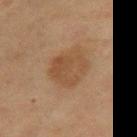biopsy status — no biopsy performed (imaged during a skin exam) | location — the right thigh | lesion diameter — ≈4.5 mm | illumination — cross-polarized | patient — female, in their 40s | automated metrics — a lesion area of about 14 mm², a shape eccentricity near 0.55, and a symmetry-axis asymmetry near 0.25; border irregularity of about 2.5 on a 0–10 scale and a within-lesion color-variation index near 3/10; lesion-presence confidence of about 100/100 | acquisition — 15 mm crop, total-body photography.